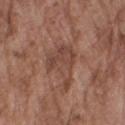biopsy_status: not biopsied; imaged during a skin examination
patient:
  sex: male
  age_approx: 75
image:
  source: total-body photography crop
  field_of_view_mm: 15
site: right upper arm